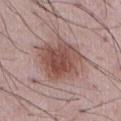Imaged during a routine full-body skin examination; the lesion was not biopsied and no histopathology is available.
A male subject aged around 55.
From the front of the torso.
Imaged with white-light lighting.
A 15 mm close-up extracted from a 3D total-body photography capture.
The total-body-photography lesion software estimated a footprint of about 22 mm², a shape eccentricity near 0.55, and a shape-asymmetry score of about 0.2 (0 = symmetric). It also reported a lesion color around L≈50 a*≈20 b*≈23 in CIELAB, roughly 12 lightness units darker than nearby skin, and a lesion-to-skin contrast of about 9 (normalized; higher = more distinct). The analysis additionally found a nevus-likeness score of about 95/100 and lesion-presence confidence of about 100/100.
About 6 mm across.